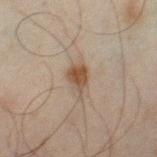Q: Was this lesion biopsied?
A: total-body-photography surveillance lesion; no biopsy
Q: What did automated image analysis measure?
A: an area of roughly 6.5 mm² and an outline eccentricity of about 0.8 (0 = round, 1 = elongated)
Q: Who is the patient?
A: male, in their mid-40s
Q: How was this image acquired?
A: 15 mm crop, total-body photography
Q: Lesion location?
A: the right thigh
Q: What lighting was used for the tile?
A: cross-polarized